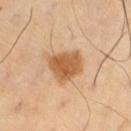Q: Was a biopsy performed?
A: imaged on a skin check; not biopsied
Q: Who is the patient?
A: male, aged 53–57
Q: What is the anatomic site?
A: the right thigh
Q: What kind of image is this?
A: total-body-photography crop, ~15 mm field of view
Q: Automated lesion metrics?
A: a lesion–skin lightness drop of about 13; a border-irregularity rating of about 2/10, a color-variation rating of about 3/10, and radial color variation of about 1
Q: How was the tile lit?
A: cross-polarized